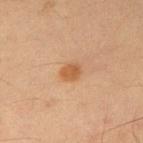follow-up: catalogued during a skin exam; not biopsied | diameter: ≈2.5 mm | body site: the arm | acquisition: 15 mm crop, total-body photography | subject: male, roughly 35 years of age | lighting: cross-polarized.